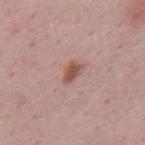Notes:
– lesion diameter — ~3 mm (longest diameter)
– image — ~15 mm tile from a whole-body skin photo
– location — the upper back
– subject — male, roughly 50 years of age
– automated lesion analysis — a footprint of about 4 mm² and a shape eccentricity near 0.8; an average lesion color of about L≈52 a*≈22 b*≈26 (CIELAB), a lesion–skin lightness drop of about 11, and a lesion-to-skin contrast of about 8.5 (normalized; higher = more distinct); a nevus-likeness score of about 80/100 and a detector confidence of about 100 out of 100 that the crop contains a lesion